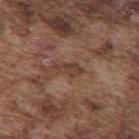Case summary:
• biopsy status — no biopsy performed (imaged during a skin exam)
• size — ~3.5 mm (longest diameter)
• patient — male, about 75 years old
• acquisition — total-body-photography crop, ~15 mm field of view
• tile lighting — white-light illumination
• body site — the back
• automated metrics — an area of roughly 3.5 mm²; an average lesion color of about L≈40 a*≈19 b*≈26 (CIELAB), about 7 CIELAB-L* units darker than the surrounding skin, and a lesion-to-skin contrast of about 6 (normalized; higher = more distinct); a border-irregularity index near 4/10, internal color variation of about 1 on a 0–10 scale, and a peripheral color-asymmetry measure near 0.5